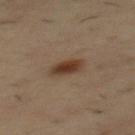The lesion was tiled from a total-body skin photograph and was not biopsied. The patient is a male about 55 years old. The tile uses cross-polarized illumination. About 3.5 mm across. The lesion is on the mid back. The lesion-visualizer software estimated a footprint of about 5.5 mm² and a symmetry-axis asymmetry near 0.15. And it measured a mean CIELAB color near L≈36 a*≈17 b*≈27 and a lesion–skin lightness drop of about 11. The analysis additionally found border irregularity of about 1.5 on a 0–10 scale, a color-variation rating of about 4/10, and radial color variation of about 1. The software also gave an automated nevus-likeness rating near 100 out of 100. A 15 mm crop from a total-body photograph taken for skin-cancer surveillance.